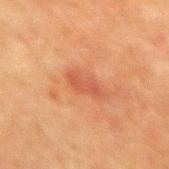<tbp_lesion>
  <biopsy_status>not biopsied; imaged during a skin examination</biopsy_status>
  <patient>
    <sex>male</sex>
    <age_approx>50</age_approx>
  </patient>
  <site>mid back</site>
  <lighting>cross-polarized</lighting>
  <image>
    <source>total-body photography crop</source>
    <field_of_view_mm>15</field_of_view_mm>
  </image>
  <automated_metrics>
    <nevus_likeness_0_100>0</nevus_likeness_0_100>
  </automated_metrics>
</tbp_lesion>